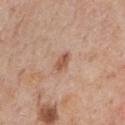A male subject, about 60 years old.
About 2.5 mm across.
Automated tile analysis of the lesion measured a lesion color around L≈55 a*≈21 b*≈31 in CIELAB, roughly 11 lightness units darker than nearby skin, and a normalized lesion–skin contrast near 7.5.
Cropped from a whole-body photographic skin survey; the tile spans about 15 mm.
Located on the chest.
The tile uses white-light illumination.